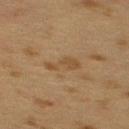Part of a total-body skin-imaging series; this lesion was reviewed on a skin check and was not flagged for biopsy. A female subject in their 40s. A roughly 15 mm field-of-view crop from a total-body skin photograph. The lesion is located on the upper back.